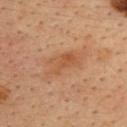No biopsy was performed on this lesion — it was imaged during a full skin examination and was not determined to be concerning.
A roughly 15 mm field-of-view crop from a total-body skin photograph.
The subject is a male in their mid- to late 30s.
Approximately 5 mm at its widest.
Imaged with cross-polarized lighting.
The lesion is on the upper back.